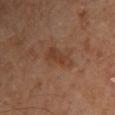<record>
<biopsy_status>not biopsied; imaged during a skin examination</biopsy_status>
<image>
  <source>total-body photography crop</source>
  <field_of_view_mm>15</field_of_view_mm>
</image>
<lesion_size>
  <long_diameter_mm_approx>4.5</long_diameter_mm_approx>
</lesion_size>
<site>chest</site>
<patient>
  <sex>female</sex>
  <age_approx>60</age_approx>
</patient>
<automated_metrics>
  <area_mm2_approx>7.0</area_mm2_approx>
  <shape_asymmetry>0.35</shape_asymmetry>
  <cielab_L>39</cielab_L>
  <cielab_a>20</cielab_a>
  <cielab_b>30</cielab_b>
  <vs_skin_darker_L>7.0</vs_skin_darker_L>
  <vs_skin_contrast_norm>6.5</vs_skin_contrast_norm>
</automated_metrics>
</record>